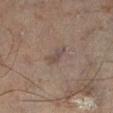notes: catalogued during a skin exam; not biopsied | location: the left lower leg | acquisition: total-body-photography crop, ~15 mm field of view | automated metrics: a lesion area of about 3.5 mm², a shape eccentricity near 0.95, and a shape-asymmetry score of about 0.3 (0 = symmetric); a border-irregularity rating of about 3.5/10, a within-lesion color-variation index near 1/10, and peripheral color asymmetry of about 0.5 | subject: male, in their mid- to late 40s.